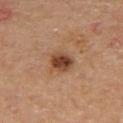The lesion was photographed on a routine skin check and not biopsied; there is no pathology result. A 15 mm close-up extracted from a 3D total-body photography capture. From the right thigh. A male patient aged around 65.